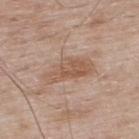{"lighting": "white-light", "automated_metrics": {"area_mm2_approx": 11.0, "nevus_likeness_0_100": 0}, "site": "upper back", "patient": {"sex": "male", "age_approx": 65}, "image": {"source": "total-body photography crop", "field_of_view_mm": 15}}